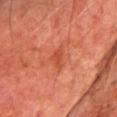Imaged during a routine full-body skin examination; the lesion was not biopsied and no histopathology is available. This image is a 15 mm lesion crop taken from a total-body photograph. The subject is a male approximately 75 years of age. From the chest.